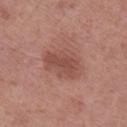notes=total-body-photography surveillance lesion; no biopsy
acquisition=15 mm crop, total-body photography
location=the right lower leg
lesion size=~5 mm (longest diameter)
image-analysis metrics=a footprint of about 13 mm² and a shape eccentricity near 0.75
tile lighting=white-light
patient=male, approximately 55 years of age